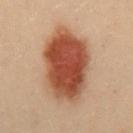The lesion was tiled from a total-body skin photograph and was not biopsied. The patient is a female aged 28–32. Located on the mid back. Automated image analysis of the tile measured a lesion color around L≈40 a*≈21 b*≈27 in CIELAB, about 14 CIELAB-L* units darker than the surrounding skin, and a normalized lesion–skin contrast near 11.5. The analysis additionally found border irregularity of about 2 on a 0–10 scale, internal color variation of about 6 on a 0–10 scale, and peripheral color asymmetry of about 2. A lesion tile, about 15 mm wide, cut from a 3D total-body photograph. The tile uses cross-polarized illumination.